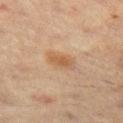• follow-up: no biopsy performed (imaged during a skin exam)
• anatomic site: the leg
• automated lesion analysis: a lesion area of about 6 mm² and two-axis asymmetry of about 0.25; a detector confidence of about 100 out of 100 that the crop contains a lesion
• patient: male, approximately 60 years of age
• image: 15 mm crop, total-body photography
• tile lighting: cross-polarized illumination